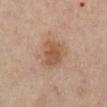| feature | finding |
|---|---|
| notes | no biopsy performed (imaged during a skin exam) |
| body site | the left lower leg |
| image-analysis metrics | a lesion area of about 10 mm² and a symmetry-axis asymmetry near 0.2; border irregularity of about 2.5 on a 0–10 scale, internal color variation of about 3.5 on a 0–10 scale, and a peripheral color-asymmetry measure near 1; a classifier nevus-likeness of about 70/100 and a lesion-detection confidence of about 100/100 |
| image | ~15 mm crop, total-body skin-cancer survey |
| subject | female, approximately 60 years of age |
| diameter | ~4 mm (longest diameter) |